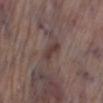The lesion was tiled from a total-body skin photograph and was not biopsied.
A close-up tile cropped from a whole-body skin photograph, about 15 mm across.
The tile uses white-light illumination.
The subject is a male aged around 70.
An algorithmic analysis of the crop reported an average lesion color of about L≈38 a*≈16 b*≈18 (CIELAB), roughly 8 lightness units darker than nearby skin, and a normalized lesion–skin contrast near 7. The analysis additionally found a border-irregularity index near 2.5/10 and a color-variation rating of about 1.5/10.
The lesion is on the leg.